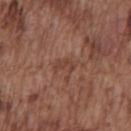Acquisition and patient details: The patient is a male aged approximately 75. From the chest. This is a white-light tile. A region of skin cropped from a whole-body photographic capture, roughly 15 mm wide. The lesion-visualizer software estimated a lesion area of about 3.5 mm², an outline eccentricity of about 0.85 (0 = round, 1 = elongated), and two-axis asymmetry of about 0.45. The software also gave about 6 CIELAB-L* units darker than the surrounding skin and a normalized lesion–skin contrast near 5. The lesion's longest dimension is about 2.5 mm.